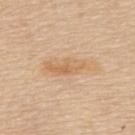Case summary:
* biopsy status — total-body-photography surveillance lesion; no biopsy
* lesion diameter — ≈6 mm
* lighting — white-light
* patient — female, aged approximately 65
* automated lesion analysis — a mean CIELAB color near L≈66 a*≈18 b*≈38, about 7 CIELAB-L* units darker than the surrounding skin, and a normalized border contrast of about 5.5; a classifier nevus-likeness of about 5/100
* imaging modality — total-body-photography crop, ~15 mm field of view
* location — the upper back